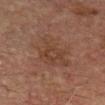Assessment: Recorded during total-body skin imaging; not selected for excision or biopsy. Context: A lesion tile, about 15 mm wide, cut from a 3D total-body photograph. Located on the head or neck. A male subject in their mid- to late 70s.